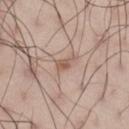The lesion was photographed on a routine skin check and not biopsied; there is no pathology result. An algorithmic analysis of the crop reported a lesion area of about 2.5 mm² and a shape eccentricity near 0.85. Located on the right thigh. A male subject, aged around 45. About 2.5 mm across. A roughly 15 mm field-of-view crop from a total-body skin photograph.